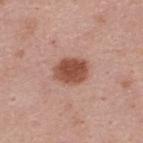{
  "biopsy_status": "not biopsied; imaged during a skin examination",
  "image": {
    "source": "total-body photography crop",
    "field_of_view_mm": 15
  },
  "site": "upper back",
  "automated_metrics": {
    "cielab_L": 50,
    "cielab_a": 24,
    "cielab_b": 29,
    "vs_skin_darker_L": 14.0,
    "vs_skin_contrast_norm": 10.5
  },
  "lighting": "white-light",
  "patient": {
    "sex": "male",
    "age_approx": 45
  },
  "lesion_size": {
    "long_diameter_mm_approx": 4.0
  }
}